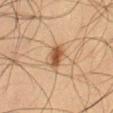Captured during whole-body skin photography for melanoma surveillance; the lesion was not biopsied. This is a cross-polarized tile. The subject is a male aged 63–67. From the right thigh. The recorded lesion diameter is about 3.5 mm. Automated image analysis of the tile measured a footprint of about 6 mm² and two-axis asymmetry of about 0.2. It also reported a lesion color around L≈45 a*≈17 b*≈30 in CIELAB and a normalized border contrast of about 8.5. The analysis additionally found a border-irregularity index near 2.5/10, a color-variation rating of about 3.5/10, and radial color variation of about 1. The software also gave a classifier nevus-likeness of about 95/100 and a lesion-detection confidence of about 100/100. This image is a 15 mm lesion crop taken from a total-body photograph.